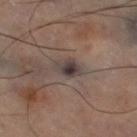Impression: The lesion was tiled from a total-body skin photograph and was not biopsied. Image and clinical context: The total-body-photography lesion software estimated a footprint of about 5.5 mm², an outline eccentricity of about 0.7 (0 = round, 1 = elongated), and a shape-asymmetry score of about 0.3 (0 = symmetric). It also reported a mean CIELAB color near L≈39 a*≈11 b*≈16, roughly 10 lightness units darker than nearby skin, and a normalized lesion–skin contrast near 10. And it measured a classifier nevus-likeness of about 0/100 and a detector confidence of about 75 out of 100 that the crop contains a lesion. This is a cross-polarized tile. A 15 mm close-up tile from a total-body photography series done for melanoma screening. The recorded lesion diameter is about 3 mm. The lesion is located on the right thigh.